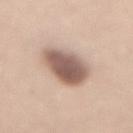Clinical impression:
Captured during whole-body skin photography for melanoma surveillance; the lesion was not biopsied.
Clinical summary:
The total-body-photography lesion software estimated a lesion area of about 17 mm², an outline eccentricity of about 0.85 (0 = round, 1 = elongated), and a shape-asymmetry score of about 0.2 (0 = symmetric). The analysis additionally found a nevus-likeness score of about 90/100 and lesion-presence confidence of about 100/100. Located on the mid back. A male patient, approximately 60 years of age. Approximately 6.5 mm at its widest. A roughly 15 mm field-of-view crop from a total-body skin photograph.